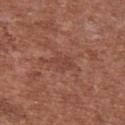workup = no biopsy performed (imaged during a skin exam); imaging modality = 15 mm crop, total-body photography; location = the chest; subject = male, aged 43–47.